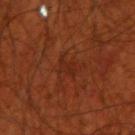Impression: No biopsy was performed on this lesion — it was imaged during a full skin examination and was not determined to be concerning. Acquisition and patient details: The lesion is on the right upper arm. Captured under cross-polarized illumination. A close-up tile cropped from a whole-body skin photograph, about 15 mm across. The patient is a male aged around 70. Longest diameter approximately 2.5 mm. Automated image analysis of the tile measured an area of roughly 2.5 mm², an eccentricity of roughly 0.9, and a symmetry-axis asymmetry near 0.3. It also reported a border-irregularity rating of about 3.5/10 and a peripheral color-asymmetry measure near 0. The analysis additionally found an automated nevus-likeness rating near 0 out of 100 and lesion-presence confidence of about 95/100.